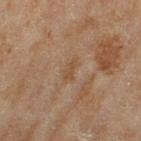This lesion was catalogued during total-body skin photography and was not selected for biopsy. A female subject aged approximately 60. About 3 mm across. Located on the left thigh. Cropped from a whole-body photographic skin survey; the tile spans about 15 mm.